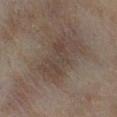{
  "biopsy_status": "not biopsied; imaged during a skin examination"
}